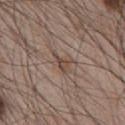follow-up: imaged on a skin check; not biopsied
size: ~3.5 mm (longest diameter)
location: the chest
tile lighting: white-light
image: total-body-photography crop, ~15 mm field of view
subject: male, aged approximately 65